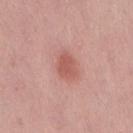Findings:
– lesion size · ≈3 mm
– image source · 15 mm crop, total-body photography
– image-analysis metrics · an area of roughly 6 mm² and a shape-asymmetry score of about 0.2 (0 = symmetric); an average lesion color of about L≈56 a*≈27 b*≈26 (CIELAB) and about 9 CIELAB-L* units darker than the surrounding skin; a within-lesion color-variation index near 1.5/10 and peripheral color asymmetry of about 0.5; a nevus-likeness score of about 95/100 and a detector confidence of about 100 out of 100 that the crop contains a lesion
– site · the right thigh
– illumination · white-light
– subject · female, approximately 45 years of age